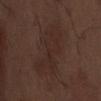Impression:
The lesion was photographed on a routine skin check and not biopsied; there is no pathology result.
Acquisition and patient details:
Located on the abdomen. A 15 mm crop from a total-body photograph taken for skin-cancer surveillance. A male patient aged approximately 70. Captured under white-light illumination. About 8 mm across.